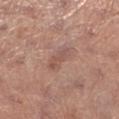* follow-up — imaged on a skin check; not biopsied
* image source — ~15 mm crop, total-body skin-cancer survey
* location — the left lower leg
* TBP lesion metrics — internal color variation of about 4.5 on a 0–10 scale and radial color variation of about 1.5
* tile lighting — white-light illumination
* subject — female, about 65 years old